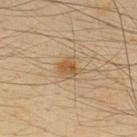patient:
  sex: male
  age_approx: 35
image:
  source: total-body photography crop
  field_of_view_mm: 15
automated_metrics:
  area_mm2_approx: 5.0
  eccentricity: 0.4
  shape_asymmetry: 0.25
  cielab_L: 48
  cielab_a: 15
  cielab_b: 33
  vs_skin_darker_L: 8.0
  vs_skin_contrast_norm: 7.0
  nevus_likeness_0_100: 80
lesion_size:
  long_diameter_mm_approx: 2.5
site: upper back